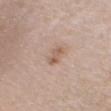The lesion was tiled from a total-body skin photograph and was not biopsied.
On the head or neck.
This image is a 15 mm lesion crop taken from a total-body photograph.
The lesion's longest dimension is about 2.5 mm.
A male patient aged around 35.